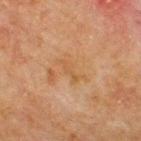Part of a total-body skin-imaging series; this lesion was reviewed on a skin check and was not flagged for biopsy.
The lesion is on the upper back.
A region of skin cropped from a whole-body photographic capture, roughly 15 mm wide.
A male patient, approximately 70 years of age.
Captured under cross-polarized illumination.